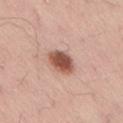Captured during whole-body skin photography for melanoma surveillance; the lesion was not biopsied.
Longest diameter approximately 3.5 mm.
This is a white-light tile.
From the back.
A lesion tile, about 15 mm wide, cut from a 3D total-body photograph.
A male patient in their mid- to late 50s.